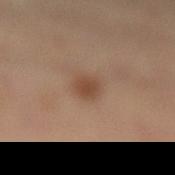{
  "biopsy_status": "not biopsied; imaged during a skin examination",
  "lesion_size": {
    "long_diameter_mm_approx": 2.5
  },
  "patient": {
    "sex": "male",
    "age_approx": 50
  },
  "automated_metrics": {
    "area_mm2_approx": 4.0,
    "eccentricity": 0.7,
    "cielab_L": 45,
    "cielab_a": 18,
    "cielab_b": 29,
    "vs_skin_darker_L": 9.0,
    "vs_skin_contrast_norm": 7.5
  },
  "image": {
    "source": "total-body photography crop",
    "field_of_view_mm": 15
  },
  "site": "left leg"
}